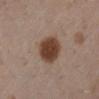This lesion was catalogued during total-body skin photography and was not selected for biopsy. The lesion is located on the left lower leg. A female patient approximately 30 years of age. The tile uses white-light illumination. A close-up tile cropped from a whole-body skin photograph, about 15 mm across. Approximately 4 mm at its widest.